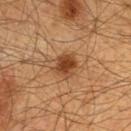Recorded during total-body skin imaging; not selected for excision or biopsy. A region of skin cropped from a whole-body photographic capture, roughly 15 mm wide. A male subject roughly 60 years of age. The lesion is on the chest.